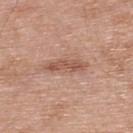  biopsy_status: not biopsied; imaged during a skin examination
  image:
    source: total-body photography crop
    field_of_view_mm: 15
  patient:
    sex: male
    age_approx: 70
  site: upper back
  lesion_size:
    long_diameter_mm_approx: 5.0
  lighting: white-light
  automated_metrics:
    area_mm2_approx: 6.5
    eccentricity: 0.95
    shape_asymmetry: 0.35
    cielab_L: 55
    cielab_a: 22
    cielab_b: 29
    vs_skin_contrast_norm: 7.0
    border_irregularity_0_10: 4.5
    color_variation_0_10: 2.0
    peripheral_color_asymmetry: 0.5
    lesion_detection_confidence_0_100: 100